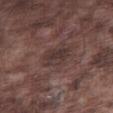The lesion was tiled from a total-body skin photograph and was not biopsied.
The lesion's longest dimension is about 3.5 mm.
An algorithmic analysis of the crop reported a footprint of about 6.5 mm², an outline eccentricity of about 0.75 (0 = round, 1 = elongated), and a shape-asymmetry score of about 0.3 (0 = symmetric). The software also gave internal color variation of about 3 on a 0–10 scale. The software also gave a classifier nevus-likeness of about 10/100 and a lesion-detection confidence of about 95/100.
On the right thigh.
Captured under white-light illumination.
The patient is a male aged 73 to 77.
A 15 mm crop from a total-body photograph taken for skin-cancer surveillance.